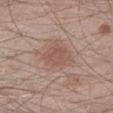Q: Is there a histopathology result?
A: no biopsy performed (imaged during a skin exam)
Q: How was the tile lit?
A: white-light
Q: How was this image acquired?
A: ~15 mm tile from a whole-body skin photo
Q: Patient demographics?
A: male, aged 33–37
Q: What is the lesion's diameter?
A: about 3.5 mm
Q: Automated lesion metrics?
A: a footprint of about 8.5 mm² and a symmetry-axis asymmetry near 0.2; a border-irregularity index near 2/10, a within-lesion color-variation index near 2/10, and peripheral color asymmetry of about 0.5; a nevus-likeness score of about 5/100
Q: What is the anatomic site?
A: the left lower leg